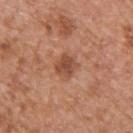follow-up = total-body-photography surveillance lesion; no biopsy | patient = male, approximately 65 years of age | acquisition = total-body-photography crop, ~15 mm field of view | location = the upper back.